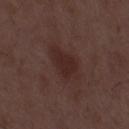| key | value |
|---|---|
| lesion size | ≈5 mm |
| automated lesion analysis | a mean CIELAB color near L≈26 a*≈18 b*≈19, about 6 CIELAB-L* units darker than the surrounding skin, and a lesion-to-skin contrast of about 7 (normalized; higher = more distinct); border irregularity of about 3 on a 0–10 scale, a within-lesion color-variation index near 2/10, and radial color variation of about 0.5 |
| illumination | white-light |
| image source | total-body-photography crop, ~15 mm field of view |
| patient | male, in their 50s |